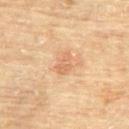Q: Was a biopsy performed?
A: catalogued during a skin exam; not biopsied
Q: What is the imaging modality?
A: 15 mm crop, total-body photography
Q: Who is the patient?
A: female, approximately 80 years of age
Q: Where on the body is the lesion?
A: the upper back
Q: Illumination type?
A: cross-polarized illumination